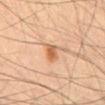• notes — catalogued during a skin exam; not biopsied
• subject — male, in their mid-30s
• body site — the front of the torso
• lesion diameter — about 2.5 mm
• tile lighting — cross-polarized
• acquisition — ~15 mm tile from a whole-body skin photo